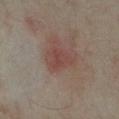The lesion is located on the leg.
The subject is a female roughly 35 years of age.
A close-up tile cropped from a whole-body skin photograph, about 15 mm across.
The lesion's longest dimension is about 3.5 mm.
This is a cross-polarized tile.